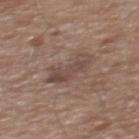<record>
<biopsy_status>not biopsied; imaged during a skin examination</biopsy_status>
<site>mid back</site>
<automated_metrics>
  <shape_asymmetry>0.45</shape_asymmetry>
  <cielab_L>46</cielab_L>
  <cielab_a>16</cielab_a>
  <cielab_b>22</cielab_b>
  <vs_skin_darker_L>8.0</vs_skin_darker_L>
  <border_irregularity_0_10>5.5</border_irregularity_0_10>
  <color_variation_0_10>2.5</color_variation_0_10>
  <peripheral_color_asymmetry>1.0</peripheral_color_asymmetry>
</automated_metrics>
<lesion_size>
  <long_diameter_mm_approx>4.5</long_diameter_mm_approx>
</lesion_size>
<lighting>white-light</lighting>
<patient>
  <sex>male</sex>
  <age_approx>65</age_approx>
</patient>
<image>
  <source>total-body photography crop</source>
  <field_of_view_mm>15</field_of_view_mm>
</image>
</record>